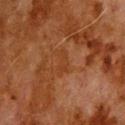Assessment: Recorded during total-body skin imaging; not selected for excision or biopsy. Context: This is a cross-polarized tile. The lesion-visualizer software estimated an automated nevus-likeness rating near 0 out of 100 and lesion-presence confidence of about 100/100. A 15 mm close-up tile from a total-body photography series done for melanoma screening. From the upper back. Longest diameter approximately 3 mm. A male patient, aged 78–82.